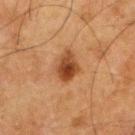• biopsy status: catalogued during a skin exam; not biopsied